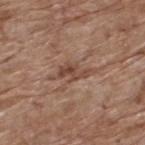• notes: imaged on a skin check; not biopsied
• lighting: white-light
• image: 15 mm crop, total-body photography
• patient: male, roughly 70 years of age
• location: the upper back